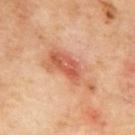<lesion>
  <biopsy_status>not biopsied; imaged during a skin examination</biopsy_status>
</lesion>